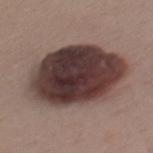A 15 mm crop from a total-body photograph taken for skin-cancer surveillance. On the mid back. Imaged with white-light lighting. The lesion's longest dimension is about 9.5 mm. A female patient, about 25 years old.